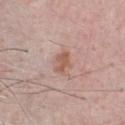notes = imaged on a skin check; not biopsied | automated lesion analysis = a within-lesion color-variation index near 2/10; a classifier nevus-likeness of about 40/100 and lesion-presence confidence of about 100/100 | subject = male, aged 48 to 52 | tile lighting = white-light illumination | anatomic site = the chest | image source = total-body-photography crop, ~15 mm field of view | size = about 3 mm.